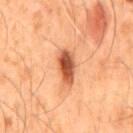Notes:
- workup — no biopsy performed (imaged during a skin exam)